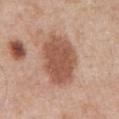Assessment:
This lesion was catalogued during total-body skin photography and was not selected for biopsy.
Acquisition and patient details:
Cropped from a total-body skin-imaging series; the visible field is about 15 mm. A male subject roughly 70 years of age. The lesion is located on the abdomen. The tile uses white-light illumination.